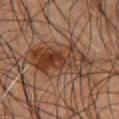Recorded during total-body skin imaging; not selected for excision or biopsy. A male patient, aged approximately 45. The lesion is on the chest. A 15 mm close-up tile from a total-body photography series done for melanoma screening.